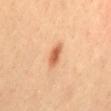Notes:
– follow-up: no biopsy performed (imaged during a skin exam)
– image: ~15 mm tile from a whole-body skin photo
– body site: the mid back
– patient: male, aged approximately 40
– automated lesion analysis: an average lesion color of about L≈64 a*≈27 b*≈39 (CIELAB), a lesion–skin lightness drop of about 13, and a normalized lesion–skin contrast near 8; a border-irregularity index near 2.5/10, a within-lesion color-variation index near 3/10, and a peripheral color-asymmetry measure near 1; an automated nevus-likeness rating near 100 out of 100 and a lesion-detection confidence of about 100/100
– lesion diameter: about 3.5 mm